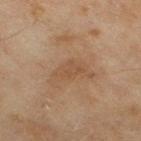Notes:
* site · the right thigh
* patient · female, about 60 years old
* tile lighting · cross-polarized
* TBP lesion metrics · a lesion area of about 3.5 mm², an eccentricity of roughly 0.85, and a symmetry-axis asymmetry near 0.45; a mean CIELAB color near L≈45 a*≈17 b*≈30, about 6 CIELAB-L* units darker than the surrounding skin, and a normalized border contrast of about 5; a border-irregularity rating of about 5/10
* imaging modality · 15 mm crop, total-body photography
* lesion diameter · ~3 mm (longest diameter)